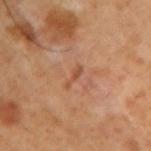Q: Automated lesion metrics?
A: an outline eccentricity of about 0.95 (0 = round, 1 = elongated); a classifier nevus-likeness of about 0/100 and a detector confidence of about 100 out of 100 that the crop contains a lesion
Q: How was this image acquired?
A: total-body-photography crop, ~15 mm field of view
Q: Where on the body is the lesion?
A: the right upper arm
Q: What are the patient's age and sex?
A: male, in their 50s
Q: What lighting was used for the tile?
A: cross-polarized